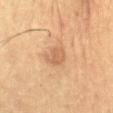Assessment:
This lesion was catalogued during total-body skin photography and was not selected for biopsy.
Acquisition and patient details:
A male patient, in their 60s. On the front of the torso. An algorithmic analysis of the crop reported a footprint of about 5.5 mm² and two-axis asymmetry of about 0.2. The analysis additionally found border irregularity of about 2 on a 0–10 scale and a color-variation rating of about 1.5/10. Imaged with cross-polarized lighting. Longest diameter approximately 3 mm. A 15 mm close-up tile from a total-body photography series done for melanoma screening.